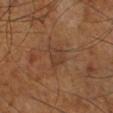The lesion was tiled from a total-body skin photograph and was not biopsied.
A male patient aged around 70.
On the right lower leg.
Automated image analysis of the tile measured an area of roughly 5.5 mm², an outline eccentricity of about 0.55 (0 = round, 1 = elongated), and a symmetry-axis asymmetry near 0.45. The software also gave border irregularity of about 4 on a 0–10 scale, a color-variation rating of about 2.5/10, and a peripheral color-asymmetry measure near 1. The software also gave a detector confidence of about 100 out of 100 that the crop contains a lesion.
A close-up tile cropped from a whole-body skin photograph, about 15 mm across.
Captured under cross-polarized illumination.
The recorded lesion diameter is about 3 mm.